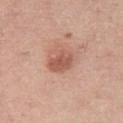The lesion was photographed on a routine skin check and not biopsied; there is no pathology result. Approximately 3.5 mm at its widest. Captured under white-light illumination. The subject is a female about 50 years old. On the left thigh. An algorithmic analysis of the crop reported an area of roughly 7 mm², a shape eccentricity near 0.7, and a symmetry-axis asymmetry near 0.2. The analysis additionally found a lesion-detection confidence of about 100/100. A 15 mm close-up extracted from a 3D total-body photography capture.